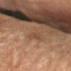follow-up = imaged on a skin check; not biopsied
size = about 2.5 mm
imaging modality = ~15 mm tile from a whole-body skin photo
site = the arm
patient = female, aged 68–72